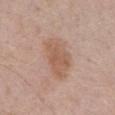Case summary:
• workup — no biopsy performed (imaged during a skin exam)
• image-analysis metrics — a lesion area of about 16 mm², an eccentricity of roughly 0.8, and a symmetry-axis asymmetry near 0.2; border irregularity of about 2.5 on a 0–10 scale and a peripheral color-asymmetry measure near 1; lesion-presence confidence of about 100/100
• anatomic site — the abdomen
• patient — male, about 70 years old
• lighting — white-light illumination
• imaging modality — ~15 mm crop, total-body skin-cancer survey